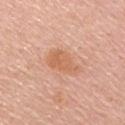Case summary:
- follow-up · no biopsy performed (imaged during a skin exam)
- automated lesion analysis · a lesion color around L≈63 a*≈24 b*≈35 in CIELAB, about 8 CIELAB-L* units darker than the surrounding skin, and a normalized lesion–skin contrast near 6.5; a nevus-likeness score of about 35/100 and a detector confidence of about 100 out of 100 that the crop contains a lesion
- image · total-body-photography crop, ~15 mm field of view
- anatomic site · the chest
- patient · male, in their mid-60s
- lighting · white-light